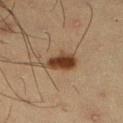Case summary:
• workup — total-body-photography surveillance lesion; no biopsy
• anatomic site — the right lower leg
• image-analysis metrics — a mean CIELAB color near L≈36 a*≈18 b*≈29, about 14 CIELAB-L* units darker than the surrounding skin, and a lesion-to-skin contrast of about 12 (normalized; higher = more distinct); a classifier nevus-likeness of about 100/100
• acquisition — ~15 mm tile from a whole-body skin photo
• tile lighting — cross-polarized
• lesion size — ~4 mm (longest diameter)
• patient — male, approximately 35 years of age